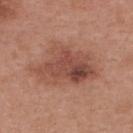Assessment: No biopsy was performed on this lesion — it was imaged during a full skin examination and was not determined to be concerning. Acquisition and patient details: A 15 mm close-up extracted from a 3D total-body photography capture. From the back. The subject is a female aged 38 to 42.